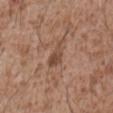Q: Was this lesion biopsied?
A: catalogued during a skin exam; not biopsied
Q: What did automated image analysis measure?
A: a classifier nevus-likeness of about 10/100 and lesion-presence confidence of about 100/100
Q: What lighting was used for the tile?
A: white-light illumination
Q: Who is the patient?
A: male, in their mid-40s
Q: What is the anatomic site?
A: the abdomen
Q: What is the imaging modality?
A: ~15 mm tile from a whole-body skin photo
Q: Lesion size?
A: about 3.5 mm